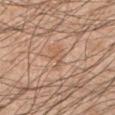No biopsy was performed on this lesion — it was imaged during a full skin examination and was not determined to be concerning.
A male patient in their 50s.
The recorded lesion diameter is about 3 mm.
A 15 mm close-up extracted from a 3D total-body photography capture.
On the left upper arm.